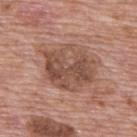Captured during whole-body skin photography for melanoma surveillance; the lesion was not biopsied. The lesion is on the upper back. The patient is a male in their 70s. A 15 mm crop from a total-body photograph taken for skin-cancer surveillance.